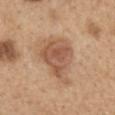Approximately 6.5 mm at its widest. A lesion tile, about 15 mm wide, cut from a 3D total-body photograph. The lesion-visualizer software estimated a mean CIELAB color near L≈55 a*≈20 b*≈32, about 11 CIELAB-L* units darker than the surrounding skin, and a lesion-to-skin contrast of about 7.5 (normalized; higher = more distinct). And it measured an automated nevus-likeness rating near 60 out of 100 and a detector confidence of about 100 out of 100 that the crop contains a lesion. This is a white-light tile. A female subject, about 60 years old. Located on the back.